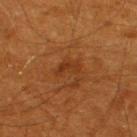Captured during whole-body skin photography for melanoma surveillance; the lesion was not biopsied. The total-body-photography lesion software estimated an outline eccentricity of about 0.85 (0 = round, 1 = elongated) and a symmetry-axis asymmetry near 0.45. And it measured a border-irregularity rating of about 5/10, a within-lesion color-variation index near 0.5/10, and radial color variation of about 0. The analysis additionally found an automated nevus-likeness rating near 0 out of 100. Captured under cross-polarized illumination. A male subject, approximately 60 years of age. A 15 mm close-up tile from a total-body photography series done for melanoma screening. From the upper back. Measured at roughly 3 mm in maximum diameter.